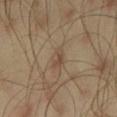| feature | finding |
|---|---|
| workup | total-body-photography surveillance lesion; no biopsy |
| patient | male, aged 43 to 47 |
| automated lesion analysis | a footprint of about 3.5 mm² and an outline eccentricity of about 0.85 (0 = round, 1 = elongated); a border-irregularity index near 3/10 and a color-variation rating of about 2/10 |
| image | 15 mm crop, total-body photography |
| location | the left thigh |